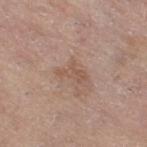workup = catalogued during a skin exam; not biopsied | lesion diameter = about 3.5 mm | anatomic site = the leg | patient = female, aged 63–67 | illumination = white-light | image source = ~15 mm crop, total-body skin-cancer survey.